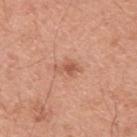Imaged during a routine full-body skin examination; the lesion was not biopsied and no histopathology is available.
The lesion is on the upper back.
The lesion-visualizer software estimated a lesion area of about 3 mm², a shape eccentricity near 0.8, and a shape-asymmetry score of about 0.4 (0 = symmetric). And it measured a lesion color around L≈57 a*≈25 b*≈32 in CIELAB and a normalized border contrast of about 6.5. It also reported a within-lesion color-variation index near 2/10 and a peripheral color-asymmetry measure near 1.
A 15 mm close-up tile from a total-body photography series done for melanoma screening.
A male patient, in their 50s.